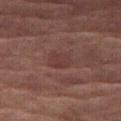Clinical impression:
No biopsy was performed on this lesion — it was imaged during a full skin examination and was not determined to be concerning.
Context:
A roughly 15 mm field-of-view crop from a total-body skin photograph. A female patient, roughly 70 years of age. Located on the leg.